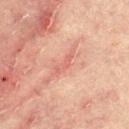Imaged during a routine full-body skin examination; the lesion was not biopsied and no histopathology is available.
From the leg.
A male patient approximately 65 years of age.
Cropped from a whole-body photographic skin survey; the tile spans about 15 mm.